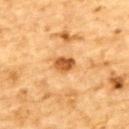Clinical impression: Captured during whole-body skin photography for melanoma surveillance; the lesion was not biopsied. Background: The lesion is located on the upper back. A 15 mm crop from a total-body photograph taken for skin-cancer surveillance. This is a cross-polarized tile. A male patient aged approximately 85 or older.